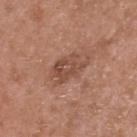A lesion tile, about 15 mm wide, cut from a 3D total-body photograph. A male patient, aged 48 to 52. The lesion is on the head or neck. Approximately 4.5 mm at its widest.